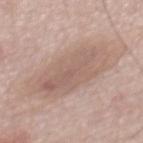This lesion was catalogued during total-body skin photography and was not selected for biopsy.
A male patient in their mid-50s.
A region of skin cropped from a whole-body photographic capture, roughly 15 mm wide.
The lesion's longest dimension is about 9 mm.
Located on the mid back.
The tile uses white-light illumination.